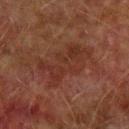– TBP lesion metrics · a lesion area of about 19 mm², an outline eccentricity of about 0.85 (0 = round, 1 = elongated), and a symmetry-axis asymmetry near 0.35; a lesion–skin lightness drop of about 5 and a normalized lesion–skin contrast near 5.5; border irregularity of about 5.5 on a 0–10 scale, a within-lesion color-variation index near 3.5/10, and radial color variation of about 1
– tile lighting · cross-polarized illumination
– location · the arm
– diameter · ≈7 mm
– subject · male, about 75 years old
– acquisition · 15 mm crop, total-body photography